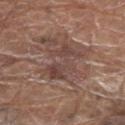Imaged during a routine full-body skin examination; the lesion was not biopsied and no histopathology is available. A 15 mm close-up tile from a total-body photography series done for melanoma screening. Imaged with white-light lighting. The lesion is on the right upper arm. An algorithmic analysis of the crop reported a border-irregularity index near 8.5/10 and internal color variation of about 4.5 on a 0–10 scale. It also reported a nevus-likeness score of about 0/100. The lesion's longest dimension is about 5.5 mm. The subject is a male aged 78–82.